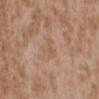follow-up: no biopsy performed (imaged during a skin exam)
patient: female, aged around 35
location: the upper back
image: ~15 mm tile from a whole-body skin photo
size: ≈2.5 mm
automated metrics: roughly 5 lightness units darker than nearby skin and a normalized border contrast of about 4.5; a detector confidence of about 100 out of 100 that the crop contains a lesion